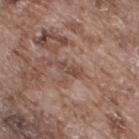Impression:
This lesion was catalogued during total-body skin photography and was not selected for biopsy.
Context:
On the right thigh. A 15 mm crop from a total-body photograph taken for skin-cancer surveillance. A male patient aged 73–77. About 3 mm across. The tile uses white-light illumination.